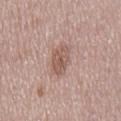From the mid back. The subject is a male aged around 55. Cropped from a whole-body photographic skin survey; the tile spans about 15 mm. The tile uses white-light illumination.